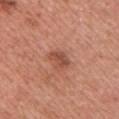workup — imaged on a skin check; not biopsied
image — ~15 mm tile from a whole-body skin photo
lighting — white-light
lesion diameter — about 3 mm
body site — the chest
subject — female, in their 40s